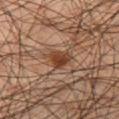| feature | finding |
|---|---|
| follow-up | total-body-photography surveillance lesion; no biopsy |
| body site | the left thigh |
| image | ~15 mm tile from a whole-body skin photo |
| subject | male, aged 43 to 47 |